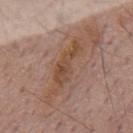Q: Is there a histopathology result?
A: imaged on a skin check; not biopsied
Q: How was the tile lit?
A: white-light illumination
Q: What is the lesion's diameter?
A: about 7 mm
Q: Who is the patient?
A: male, aged approximately 60
Q: Where on the body is the lesion?
A: the mid back
Q: What kind of image is this?
A: total-body-photography crop, ~15 mm field of view